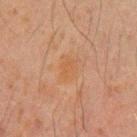image source: ~15 mm crop, total-body skin-cancer survey
subject: male, roughly 35 years of age
illumination: cross-polarized
diameter: about 3 mm
automated lesion analysis: an eccentricity of roughly 0.7 and a shape-asymmetry score of about 0.25 (0 = symmetric); a lesion–skin lightness drop of about 3
location: the left upper arm